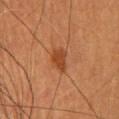This lesion was catalogued during total-body skin photography and was not selected for biopsy. Imaged with cross-polarized lighting. The recorded lesion diameter is about 3.5 mm. A 15 mm close-up tile from a total-body photography series done for melanoma screening. A female patient aged 58–62. The lesion is located on the head or neck.